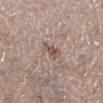notes=catalogued during a skin exam; not biopsied
acquisition=~15 mm tile from a whole-body skin photo
subject=male, aged around 65
anatomic site=the left lower leg
lighting=white-light illumination
size=~3 mm (longest diameter)